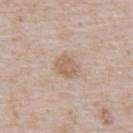Impression:
Imaged during a routine full-body skin examination; the lesion was not biopsied and no histopathology is available.
Acquisition and patient details:
The subject is a male aged approximately 80. A roughly 15 mm field-of-view crop from a total-body skin photograph. On the abdomen. Imaged with white-light lighting.